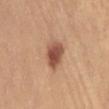subject: female, in their mid- to late 20s
imaging modality: total-body-photography crop, ~15 mm field of view
site: the abdomen